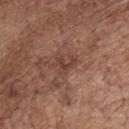workup: imaged on a skin check; not biopsied
location: the chest
image source: ~15 mm crop, total-body skin-cancer survey
patient: male, aged 68–72
tile lighting: white-light illumination
image-analysis metrics: a lesion area of about 4 mm², an eccentricity of roughly 0.75, and two-axis asymmetry of about 0.5; a lesion color around L≈41 a*≈20 b*≈26 in CIELAB, roughly 8 lightness units darker than nearby skin, and a lesion-to-skin contrast of about 6.5 (normalized; higher = more distinct); an automated nevus-likeness rating near 0 out of 100 and a detector confidence of about 95 out of 100 that the crop contains a lesion
lesion size: ≈3.5 mm